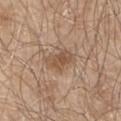| field | value |
|---|---|
| lesion diameter | ≈4 mm |
| lighting | white-light illumination |
| patient | male, roughly 65 years of age |
| location | the left upper arm |
| image | ~15 mm tile from a whole-body skin photo |